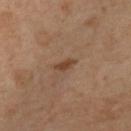The lesion was tiled from a total-body skin photograph and was not biopsied.
This is a cross-polarized tile.
The patient is a female aged 48 to 52.
A 15 mm crop from a total-body photograph taken for skin-cancer surveillance.
From the left lower leg.
An algorithmic analysis of the crop reported a footprint of about 2.5 mm², an eccentricity of roughly 0.9, and a symmetry-axis asymmetry near 0.3. The analysis additionally found an average lesion color of about L≈43 a*≈20 b*≈31 (CIELAB), roughly 9 lightness units darker than nearby skin, and a normalized border contrast of about 8. The software also gave a border-irregularity index near 3/10, a color-variation rating of about 0/10, and peripheral color asymmetry of about 0.